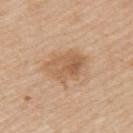Q: Is there a histopathology result?
A: no biopsy performed (imaged during a skin exam)
Q: What is the anatomic site?
A: the upper back
Q: What lighting was used for the tile?
A: white-light
Q: What is the imaging modality?
A: 15 mm crop, total-body photography
Q: What did automated image analysis measure?
A: a border-irregularity rating of about 2.5/10, internal color variation of about 5.5 on a 0–10 scale, and peripheral color asymmetry of about 2
Q: Lesion size?
A: about 5.5 mm
Q: Patient demographics?
A: male, roughly 65 years of age